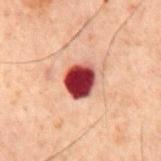Findings:
- follow-up: catalogued during a skin exam; not biopsied
- acquisition: total-body-photography crop, ~15 mm field of view
- tile lighting: cross-polarized
- subject: male, about 60 years old
- diameter: ≈4 mm
- location: the mid back